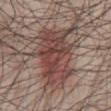Recorded during total-body skin imaging; not selected for excision or biopsy.
A male subject, about 50 years old.
The lesion is located on the abdomen.
A close-up tile cropped from a whole-body skin photograph, about 15 mm across.